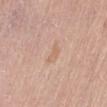Notes:
* biopsy status — imaged on a skin check; not biopsied
* subject — female, approximately 65 years of age
* image source — ~15 mm tile from a whole-body skin photo
* site — the left thigh